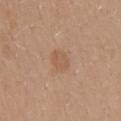The lesion was photographed on a routine skin check and not biopsied; there is no pathology result.
The lesion is located on the mid back.
Automated tile analysis of the lesion measured an area of roughly 5 mm² and two-axis asymmetry of about 0.2.
Measured at roughly 3 mm in maximum diameter.
This image is a 15 mm lesion crop taken from a total-body photograph.
The tile uses white-light illumination.
The patient is a female aged 38 to 42.